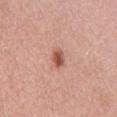subject=female, about 70 years old | site=the abdomen | image source=15 mm crop, total-body photography | lesion diameter=~2.5 mm (longest diameter).